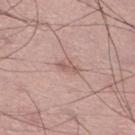Part of a total-body skin-imaging series; this lesion was reviewed on a skin check and was not flagged for biopsy. A roughly 15 mm field-of-view crop from a total-body skin photograph. Automated tile analysis of the lesion measured a mean CIELAB color near L≈58 a*≈20 b*≈24, roughly 9 lightness units darker than nearby skin, and a normalized border contrast of about 6. The analysis additionally found a border-irregularity rating of about 3.5/10 and a peripheral color-asymmetry measure near 0. And it measured an automated nevus-likeness rating near 0 out of 100 and a detector confidence of about 100 out of 100 that the crop contains a lesion. A male patient approximately 50 years of age. The lesion is on the right lower leg.